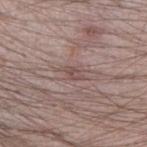This lesion was catalogued during total-body skin photography and was not selected for biopsy.
A male subject, in their mid- to late 20s.
From the right forearm.
Automated tile analysis of the lesion measured roughly 7 lightness units darker than nearby skin and a normalized border contrast of about 5.5. It also reported a border-irregularity rating of about 4/10, internal color variation of about 3 on a 0–10 scale, and radial color variation of about 1.5. The analysis additionally found a nevus-likeness score of about 0/100.
About 2.5 mm across.
A close-up tile cropped from a whole-body skin photograph, about 15 mm across.